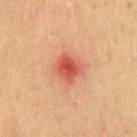{"biopsy_status": "not biopsied; imaged during a skin examination"}